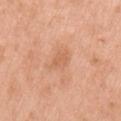biopsy_status: not biopsied; imaged during a skin examination
lesion_size:
  long_diameter_mm_approx: 3.0
site: left upper arm
patient:
  sex: female
  age_approx: 70
image:
  source: total-body photography crop
  field_of_view_mm: 15
lighting: white-light